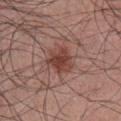lesion_size:
  long_diameter_mm_approx: 3.0
site: chest
patient:
  sex: male
  age_approx: 40
image:
  source: total-body photography crop
  field_of_view_mm: 15